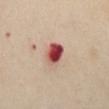Q: Is there a histopathology result?
A: catalogued during a skin exam; not biopsied
Q: What are the patient's age and sex?
A: female, aged 53 to 57
Q: How was the tile lit?
A: cross-polarized
Q: What is the anatomic site?
A: the abdomen
Q: How large is the lesion?
A: ≈3 mm
Q: What is the imaging modality?
A: ~15 mm tile from a whole-body skin photo